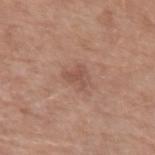Impression: Captured during whole-body skin photography for melanoma surveillance; the lesion was not biopsied. Background: Captured under white-light illumination. Approximately 2.5 mm at its widest. A male patient about 60 years old. Located on the left upper arm. Cropped from a whole-body photographic skin survey; the tile spans about 15 mm.